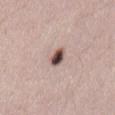{"biopsy_status": "not biopsied; imaged during a skin examination", "automated_metrics": {"eccentricity": 0.65, "vs_skin_darker_L": 20.0, "border_irregularity_0_10": 1.5, "color_variation_0_10": 8.5, "peripheral_color_asymmetry": 2.5, "nevus_likeness_0_100": 100}, "image": {"source": "total-body photography crop", "field_of_view_mm": 15}, "patient": {"sex": "female", "age_approx": 35}, "lesion_size": {"long_diameter_mm_approx": 2.5}, "site": "abdomen"}